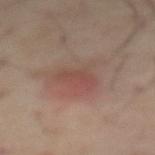Case summary:
- biopsy status · catalogued during a skin exam; not biopsied
- illumination · cross-polarized
- patient · male, about 65 years old
- imaging modality · 15 mm crop, total-body photography
- site · the abdomen
- diameter · ≈3 mm